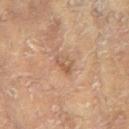Imaged during a routine full-body skin examination; the lesion was not biopsied and no histopathology is available. On the right arm. A female patient, aged 78–82. The lesion's longest dimension is about 2.5 mm. This image is a 15 mm lesion crop taken from a total-body photograph. The total-body-photography lesion software estimated an area of roughly 3.5 mm² and a shape-asymmetry score of about 0.4 (0 = symmetric). And it measured about 7 CIELAB-L* units darker than the surrounding skin and a normalized border contrast of about 5.5. And it measured a color-variation rating of about 3.5/10 and peripheral color asymmetry of about 1. The analysis additionally found a classifier nevus-likeness of about 0/100 and lesion-presence confidence of about 100/100.